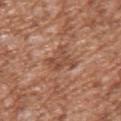A region of skin cropped from a whole-body photographic capture, roughly 15 mm wide. The patient is a male about 45 years old. This is a white-light tile. From the arm. An algorithmic analysis of the crop reported an area of roughly 7.5 mm², an outline eccentricity of about 0.65 (0 = round, 1 = elongated), and two-axis asymmetry of about 0.4. And it measured a border-irregularity rating of about 5/10. Longest diameter approximately 4 mm.